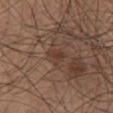A male subject, aged 73–77. This is a white-light tile. A 15 mm close-up extracted from a 3D total-body photography capture. The lesion is located on the front of the torso. Approximately 2.5 mm at its widest.